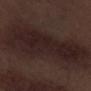Assessment: Imaged during a routine full-body skin examination; the lesion was not biopsied and no histopathology is available. Image and clinical context: The patient is a male roughly 70 years of age. The lesion is on the right lower leg. Longest diameter approximately 12.5 mm. Captured under white-light illumination. A lesion tile, about 15 mm wide, cut from a 3D total-body photograph.